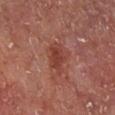This lesion was catalogued during total-body skin photography and was not selected for biopsy.
The subject is a male aged around 65.
Approximately 3.5 mm at its widest.
The tile uses cross-polarized illumination.
The lesion is on the leg.
A region of skin cropped from a whole-body photographic capture, roughly 15 mm wide.